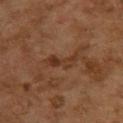Captured during whole-body skin photography for melanoma surveillance; the lesion was not biopsied. The tile uses cross-polarized illumination. The lesion's longest dimension is about 4 mm. An algorithmic analysis of the crop reported a classifier nevus-likeness of about 0/100. A female patient, aged approximately 60. Cropped from a total-body skin-imaging series; the visible field is about 15 mm. From the upper back.